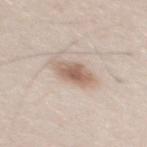Imaged during a routine full-body skin examination; the lesion was not biopsied and no histopathology is available. A 15 mm close-up extracted from a 3D total-body photography capture. Longest diameter approximately 4 mm. Imaged with white-light lighting. Automated image analysis of the tile measured a nevus-likeness score of about 95/100 and a lesion-detection confidence of about 100/100. A male subject aged 33 to 37. The lesion is located on the mid back.